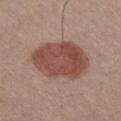Captured during whole-body skin photography for melanoma surveillance; the lesion was not biopsied.
The lesion is on the right upper arm.
A male patient in their mid- to late 50s.
A close-up tile cropped from a whole-body skin photograph, about 15 mm across.